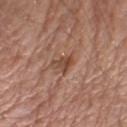This lesion was catalogued during total-body skin photography and was not selected for biopsy.
A 15 mm close-up extracted from a 3D total-body photography capture.
An algorithmic analysis of the crop reported a nevus-likeness score of about 0/100 and a detector confidence of about 100 out of 100 that the crop contains a lesion.
The recorded lesion diameter is about 3 mm.
The lesion is located on the left upper arm.
A male subject, in their mid-60s.